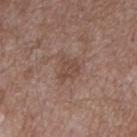Case summary:
– workup — no biopsy performed (imaged during a skin exam)
– imaging modality — 15 mm crop, total-body photography
– patient — male, aged approximately 50
– anatomic site — the right forearm
– tile lighting — white-light
– diameter — ≈3 mm